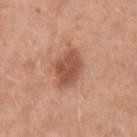The lesion was photographed on a routine skin check and not biopsied; there is no pathology result.
The tile uses white-light illumination.
A female subject aged 53 to 57.
A 15 mm crop from a total-body photograph taken for skin-cancer surveillance.
From the left upper arm.
The recorded lesion diameter is about 4 mm.
The lesion-visualizer software estimated border irregularity of about 2.5 on a 0–10 scale, internal color variation of about 3 on a 0–10 scale, and radial color variation of about 1. It also reported a nevus-likeness score of about 50/100.